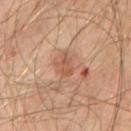– site: the front of the torso
– image: ~15 mm crop, total-body skin-cancer survey
– lesion diameter: ≈3 mm
– tile lighting: cross-polarized illumination
– TBP lesion metrics: a lesion color around L≈53 a*≈22 b*≈31 in CIELAB, roughly 9 lightness units darker than nearby skin, and a lesion-to-skin contrast of about 6 (normalized; higher = more distinct); border irregularity of about 3.5 on a 0–10 scale, a within-lesion color-variation index near 2.5/10, and a peripheral color-asymmetry measure near 1; a classifier nevus-likeness of about 0/100 and lesion-presence confidence of about 100/100
– subject: male, about 65 years old